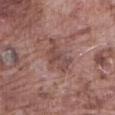Imaged during a routine full-body skin examination; the lesion was not biopsied and no histopathology is available. A roughly 15 mm field-of-view crop from a total-body skin photograph. Imaged with white-light lighting. A male subject in their mid- to late 70s. Located on the abdomen. The recorded lesion diameter is about 4.5 mm.